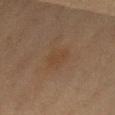Findings:
- workup · catalogued during a skin exam; not biopsied
- location · the right thigh
- image · 15 mm crop, total-body photography
- size · ≈3 mm
- subject · female, roughly 40 years of age
- illumination · cross-polarized